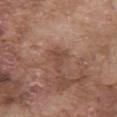Clinical summary: On the abdomen. A male patient in their mid-70s. A 15 mm crop from a total-body photograph taken for skin-cancer surveillance.